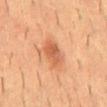Clinical impression: Imaged during a routine full-body skin examination; the lesion was not biopsied and no histopathology is available. Context: The tile uses cross-polarized illumination. On the mid back. A male subject aged around 55. A roughly 15 mm field-of-view crop from a total-body skin photograph.